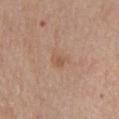| field | value |
|---|---|
| biopsy status | total-body-photography surveillance lesion; no biopsy |
| body site | the front of the torso |
| TBP lesion metrics | a lesion color around L≈56 a*≈19 b*≈30 in CIELAB and a normalized border contrast of about 5; a border-irregularity index near 3/10, a within-lesion color-variation index near 3/10, and radial color variation of about 1; a nevus-likeness score of about 0/100 and a lesion-detection confidence of about 100/100 |
| patient | male, aged around 80 |
| acquisition | 15 mm crop, total-body photography |
| lesion size | about 2.5 mm |